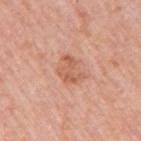biopsy status = catalogued during a skin exam; not biopsied | lesion size = about 3.5 mm | location = the right upper arm | subject = male, roughly 80 years of age | image source = 15 mm crop, total-body photography.